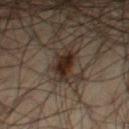Impression:
Recorded during total-body skin imaging; not selected for excision or biopsy.
Clinical summary:
Cropped from a total-body skin-imaging series; the visible field is about 15 mm. A male patient about 50 years old. The lesion-visualizer software estimated internal color variation of about 2.5 on a 0–10 scale and radial color variation of about 1. The analysis additionally found a classifier nevus-likeness of about 90/100. Approximately 4 mm at its widest. This is a cross-polarized tile. On the right thigh.